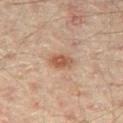Part of a total-body skin-imaging series; this lesion was reviewed on a skin check and was not flagged for biopsy.
The total-body-photography lesion software estimated a lesion color around L≈47 a*≈19 b*≈28 in CIELAB and a normalized lesion–skin contrast near 8.
A male patient, aged around 45.
A 15 mm close-up tile from a total-body photography series done for melanoma screening.
The lesion's longest dimension is about 3 mm.
This is a cross-polarized tile.
On the right thigh.